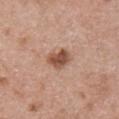{"biopsy_status": "not biopsied; imaged during a skin examination", "lesion_size": {"long_diameter_mm_approx": 3.0}, "image": {"source": "total-body photography crop", "field_of_view_mm": 15}, "site": "chest", "patient": {"sex": "male", "age_approx": 50}}